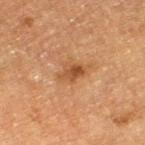Recorded during total-body skin imaging; not selected for excision or biopsy. The tile uses cross-polarized illumination. Located on the leg. The total-body-photography lesion software estimated a border-irregularity index near 3/10, a within-lesion color-variation index near 4/10, and peripheral color asymmetry of about 1.5. The software also gave an automated nevus-likeness rating near 70 out of 100. Measured at roughly 3 mm in maximum diameter. A male subject aged approximately 75. A region of skin cropped from a whole-body photographic capture, roughly 15 mm wide.